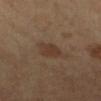The lesion was tiled from a total-body skin photograph and was not biopsied. A 15 mm close-up extracted from a 3D total-body photography capture. Measured at roughly 3 mm in maximum diameter. The patient is a female approximately 60 years of age. An algorithmic analysis of the crop reported a footprint of about 5.5 mm², an outline eccentricity of about 0.65 (0 = round, 1 = elongated), and two-axis asymmetry of about 0.25. The software also gave a lesion color around L≈33 a*≈15 b*≈25 in CIELAB, a lesion–skin lightness drop of about 6, and a lesion-to-skin contrast of about 6.5 (normalized; higher = more distinct). This is a cross-polarized tile. Located on the left lower leg.